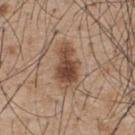<lesion>
  <site>upper back</site>
  <image>
    <source>total-body photography crop</source>
    <field_of_view_mm>15</field_of_view_mm>
  </image>
  <patient>
    <sex>male</sex>
    <age_approx>55</age_approx>
  </patient>
</lesion>